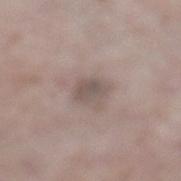Impression: Recorded during total-body skin imaging; not selected for excision or biopsy. Clinical summary: The lesion is located on the left lower leg. Cropped from a whole-body photographic skin survey; the tile spans about 15 mm. Imaged with white-light lighting. Automated tile analysis of the lesion measured a mean CIELAB color near L≈52 a*≈12 b*≈19 and roughly 9 lightness units darker than nearby skin. And it measured border irregularity of about 2.5 on a 0–10 scale and a color-variation rating of about 2.5/10. And it measured a nevus-likeness score of about 0/100. A male subject, aged approximately 65. Measured at roughly 2.5 mm in maximum diameter.